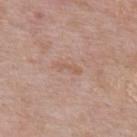No biopsy was performed on this lesion — it was imaged during a full skin examination and was not determined to be concerning. The lesion's longest dimension is about 3 mm. The tile uses white-light illumination. A 15 mm close-up tile from a total-body photography series done for melanoma screening. Located on the upper back. A male subject, in their mid- to late 50s. The lesion-visualizer software estimated an outline eccentricity of about 0.95 (0 = round, 1 = elongated) and two-axis asymmetry of about 0.25. And it measured border irregularity of about 3.5 on a 0–10 scale, a color-variation rating of about 0/10, and a peripheral color-asymmetry measure near 0.